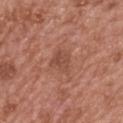Clinical impression:
No biopsy was performed on this lesion — it was imaged during a full skin examination and was not determined to be concerning.
Context:
The tile uses white-light illumination. This image is a 15 mm lesion crop taken from a total-body photograph. The recorded lesion diameter is about 2.5 mm. The lesion is on the upper back. An algorithmic analysis of the crop reported a lesion color around L≈48 a*≈23 b*≈28 in CIELAB and a normalized lesion–skin contrast near 5.5. It also reported a color-variation rating of about 2/10 and a peripheral color-asymmetry measure near 1. The subject is a female aged around 60.